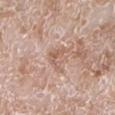A male subject, in their 60s. Cropped from a total-body skin-imaging series; the visible field is about 15 mm. The lesion is located on the left lower leg.